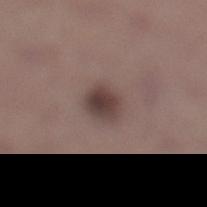Assessment: This lesion was catalogued during total-body skin photography and was not selected for biopsy. Clinical summary: The recorded lesion diameter is about 3 mm. The total-body-photography lesion software estimated a lesion area of about 6 mm², an outline eccentricity of about 0.6 (0 = round, 1 = elongated), and a shape-asymmetry score of about 0.2 (0 = symmetric). It also reported an average lesion color of about L≈40 a*≈15 b*≈18 (CIELAB), a lesion–skin lightness drop of about 12, and a lesion-to-skin contrast of about 9.5 (normalized; higher = more distinct). And it measured a border-irregularity index near 1.5/10, a color-variation rating of about 4.5/10, and a peripheral color-asymmetry measure near 1.5. The software also gave an automated nevus-likeness rating near 85 out of 100 and lesion-presence confidence of about 100/100. On the left lower leg. Cropped from a total-body skin-imaging series; the visible field is about 15 mm. A male subject, aged 43–47.